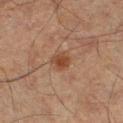{
  "biopsy_status": "not biopsied; imaged during a skin examination",
  "patient": {
    "sex": "male",
    "age_approx": 65
  },
  "automated_metrics": {
    "area_mm2_approx": 4.5,
    "shape_asymmetry": 0.25,
    "border_irregularity_0_10": 2.0,
    "color_variation_0_10": 3.0,
    "peripheral_color_asymmetry": 1.0
  },
  "site": "right lower leg",
  "image": {
    "source": "total-body photography crop",
    "field_of_view_mm": 15
  },
  "lighting": "cross-polarized",
  "lesion_size": {
    "long_diameter_mm_approx": 2.5
  }
}